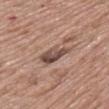Impression: The lesion was photographed on a routine skin check and not biopsied; there is no pathology result. Clinical summary: This image is a 15 mm lesion crop taken from a total-body photograph. A male patient in their 70s. Automated tile analysis of the lesion measured an area of roughly 7 mm² and an eccentricity of roughly 0.75. The analysis additionally found a lesion color around L≈48 a*≈18 b*≈24 in CIELAB, a lesion–skin lightness drop of about 13, and a normalized lesion–skin contrast near 9. The software also gave a within-lesion color-variation index near 6/10 and radial color variation of about 2. The software also gave a classifier nevus-likeness of about 0/100. About 3.5 mm across. This is a white-light tile. Located on the left upper arm.